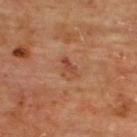No biopsy was performed on this lesion — it was imaged during a full skin examination and was not determined to be concerning. A region of skin cropped from a whole-body photographic capture, roughly 15 mm wide. The subject is a male in their mid- to late 60s. An algorithmic analysis of the crop reported a lesion area of about 3.5 mm², an eccentricity of roughly 0.8, and a symmetry-axis asymmetry near 0.35. The analysis additionally found border irregularity of about 3.5 on a 0–10 scale and a color-variation rating of about 2/10. On the upper back.